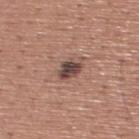Part of a total-body skin-imaging series; this lesion was reviewed on a skin check and was not flagged for biopsy.
A roughly 15 mm field-of-view crop from a total-body skin photograph.
The lesion is on the upper back.
A male patient in their mid- to late 40s.
The lesion's longest dimension is about 3 mm.
The tile uses white-light illumination.